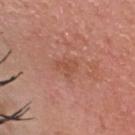The lesion was photographed on a routine skin check and not biopsied; there is no pathology result. The lesion-visualizer software estimated an outline eccentricity of about 0.55 (0 = round, 1 = elongated) and a shape-asymmetry score of about 0.4 (0 = symmetric). And it measured a border-irregularity rating of about 4/10, internal color variation of about 1 on a 0–10 scale, and peripheral color asymmetry of about 0.5. The analysis additionally found a classifier nevus-likeness of about 0/100 and a detector confidence of about 100 out of 100 that the crop contains a lesion. Measured at roughly 3 mm in maximum diameter. The lesion is located on the head or neck. Cropped from a total-body skin-imaging series; the visible field is about 15 mm. The subject is a female aged approximately 45. Captured under white-light illumination.